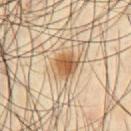Findings:
• biopsy status · no biopsy performed (imaged during a skin exam)
• body site · the chest
• patient · male, about 55 years old
• illumination · cross-polarized illumination
• image · total-body-photography crop, ~15 mm field of view
• size · ~3.5 mm (longest diameter)